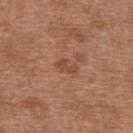Assessment:
Imaged during a routine full-body skin examination; the lesion was not biopsied and no histopathology is available.
Image and clinical context:
The lesion is located on the upper back. This image is a 15 mm lesion crop taken from a total-body photograph. The subject is a female aged approximately 40.